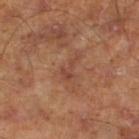Captured during whole-body skin photography for melanoma surveillance; the lesion was not biopsied. The total-body-photography lesion software estimated a footprint of about 3 mm², an eccentricity of roughly 0.9, and a symmetry-axis asymmetry near 0.65. The software also gave border irregularity of about 7.5 on a 0–10 scale, internal color variation of about 0 on a 0–10 scale, and peripheral color asymmetry of about 0. The analysis additionally found a nevus-likeness score of about 0/100 and a detector confidence of about 100 out of 100 that the crop contains a lesion. A close-up tile cropped from a whole-body skin photograph, about 15 mm across. This is a cross-polarized tile. A male subject approximately 45 years of age. The lesion is located on the leg. Measured at roughly 3 mm in maximum diameter.